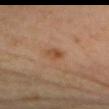Q: Was a biopsy performed?
A: no biopsy performed (imaged during a skin exam)
Q: How was the tile lit?
A: cross-polarized illumination
Q: What is the lesion's diameter?
A: ≈2.5 mm
Q: Where on the body is the lesion?
A: the chest
Q: What is the imaging modality?
A: 15 mm crop, total-body photography
Q: What are the patient's age and sex?
A: female, roughly 50 years of age